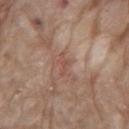{
  "biopsy_status": "not biopsied; imaged during a skin examination",
  "lesion_size": {
    "long_diameter_mm_approx": 2.5
  },
  "automated_metrics": {
    "area_mm2_approx": 2.5,
    "eccentricity": 0.85,
    "shape_asymmetry": 0.45,
    "lesion_detection_confidence_0_100": 95
  },
  "image": {
    "source": "total-body photography crop",
    "field_of_view_mm": 15
  },
  "site": "mid back",
  "lighting": "white-light",
  "patient": {
    "sex": "male",
    "age_approx": 70
  }
}